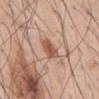<lesion>
  <biopsy_status>not biopsied; imaged during a skin examination</biopsy_status>
  <automated_metrics>
    <nevus_likeness_0_100>95</nevus_likeness_0_100>
    <lesion_detection_confidence_0_100>100</lesion_detection_confidence_0_100>
  </automated_metrics>
  <image>
    <source>total-body photography crop</source>
    <field_of_view_mm>15</field_of_view_mm>
  </image>
  <lesion_size>
    <long_diameter_mm_approx>3.0</long_diameter_mm_approx>
  </lesion_size>
  <site>abdomen</site>
  <patient>
    <sex>male</sex>
    <age_approx>45</age_approx>
  </patient>
  <lighting>white-light</lighting>
</lesion>